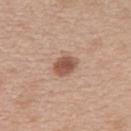<lesion>
  <biopsy_status>not biopsied; imaged during a skin examination</biopsy_status>
  <patient>
    <sex>female</sex>
    <age_approx>40</age_approx>
  </patient>
  <site>right upper arm</site>
  <lesion_size>
    <long_diameter_mm_approx>3.0</long_diameter_mm_approx>
  </lesion_size>
  <image>
    <source>total-body photography crop</source>
    <field_of_view_mm>15</field_of_view_mm>
  </image>
</lesion>